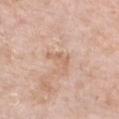Q: Was a biopsy performed?
A: catalogued during a skin exam; not biopsied
Q: What are the patient's age and sex?
A: female, aged approximately 75
Q: What is the imaging modality?
A: total-body-photography crop, ~15 mm field of view
Q: Lesion size?
A: about 3 mm
Q: Illumination type?
A: white-light
Q: Lesion location?
A: the chest
Q: What did automated image analysis measure?
A: a mean CIELAB color near L≈64 a*≈20 b*≈30, about 7 CIELAB-L* units darker than the surrounding skin, and a normalized border contrast of about 5; border irregularity of about 7.5 on a 0–10 scale, a within-lesion color-variation index near 0/10, and a peripheral color-asymmetry measure near 0